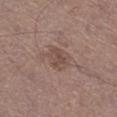{
  "lesion_size": {
    "long_diameter_mm_approx": 3.0
  },
  "site": "leg",
  "lighting": "white-light",
  "patient": {
    "sex": "male",
    "age_approx": 65
  },
  "image": {
    "source": "total-body photography crop",
    "field_of_view_mm": 15
  }
}